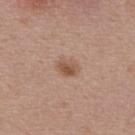Assessment: The lesion was tiled from a total-body skin photograph and was not biopsied. Background: A roughly 15 mm field-of-view crop from a total-body skin photograph. Located on the back. A female patient roughly 45 years of age. Imaged with white-light lighting.